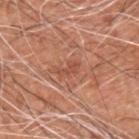* notes: no biopsy performed (imaged during a skin exam)
* size: about 3 mm
* subject: male, aged 58–62
* image: total-body-photography crop, ~15 mm field of view
* TBP lesion metrics: an area of roughly 2.5 mm² and a shape-asymmetry score of about 0.4 (0 = symmetric); an automated nevus-likeness rating near 0 out of 100 and a detector confidence of about 85 out of 100 that the crop contains a lesion
* anatomic site: the upper back